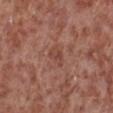Part of a total-body skin-imaging series; this lesion was reviewed on a skin check and was not flagged for biopsy.
Cropped from a whole-body photographic skin survey; the tile spans about 15 mm.
The patient is a male approximately 55 years of age.
The lesion is on the left lower leg.
The lesion's longest dimension is about 2.5 mm.